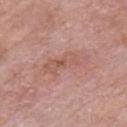This lesion was catalogued during total-body skin photography and was not selected for biopsy. The tile uses white-light illumination. About 5.5 mm across. The lesion-visualizer software estimated a border-irregularity index near 6/10, internal color variation of about 4.5 on a 0–10 scale, and peripheral color asymmetry of about 1.5. The analysis additionally found lesion-presence confidence of about 100/100. From the chest. A 15 mm close-up extracted from a 3D total-body photography capture. The patient is a male about 55 years old.